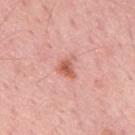This lesion was catalogued during total-body skin photography and was not selected for biopsy. A 15 mm crop from a total-body photograph taken for skin-cancer surveillance. A male patient, in their mid-60s. Measured at roughly 2.5 mm in maximum diameter. Automated tile analysis of the lesion measured border irregularity of about 4 on a 0–10 scale and a peripheral color-asymmetry measure near 1.5. And it measured lesion-presence confidence of about 100/100. Located on the mid back. Captured under white-light illumination.